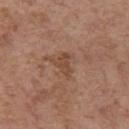Clinical summary: The lesion-visualizer software estimated an area of roughly 4.5 mm², an eccentricity of roughly 0.8, and a shape-asymmetry score of about 0.45 (0 = symmetric). It also reported a lesion color around L≈46 a*≈20 b*≈29 in CIELAB and a normalized border contrast of about 6. And it measured a border-irregularity index near 5/10 and a within-lesion color-variation index near 1/10. The patient is a female aged 63–67. The lesion is located on the left upper arm. Captured under white-light illumination. Approximately 3 mm at its widest. A region of skin cropped from a whole-body photographic capture, roughly 15 mm wide.